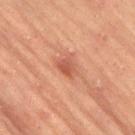follow-up=no biopsy performed (imaged during a skin exam); image source=total-body-photography crop, ~15 mm field of view; subject=female, approximately 60 years of age; anatomic site=the leg; size=about 3 mm; lighting=cross-polarized.